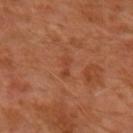Measured at roughly 3 mm in maximum diameter. Automated image analysis of the tile measured a footprint of about 2.5 mm² and a shape-asymmetry score of about 0.35 (0 = symmetric). The software also gave a lesion–skin lightness drop of about 6 and a normalized border contrast of about 5. The software also gave a color-variation rating of about 0/10 and a peripheral color-asymmetry measure near 0. The subject is a male aged around 30. This is a cross-polarized tile. Cropped from a total-body skin-imaging series; the visible field is about 15 mm. On the left forearm.